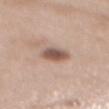Q: Is there a histopathology result?
A: imaged on a skin check; not biopsied
Q: What kind of image is this?
A: 15 mm crop, total-body photography
Q: Lesion location?
A: the mid back
Q: Patient demographics?
A: female, in their 40s
Q: Automated lesion metrics?
A: a shape eccentricity near 0.75 and a shape-asymmetry score of about 0.2 (0 = symmetric); a mean CIELAB color near L≈55 a*≈18 b*≈24 and a lesion-to-skin contrast of about 9 (normalized; higher = more distinct); an automated nevus-likeness rating near 95 out of 100 and a lesion-detection confidence of about 100/100
Q: How was the tile lit?
A: white-light illumination